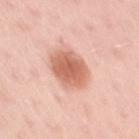Captured during whole-body skin photography for melanoma surveillance; the lesion was not biopsied.
Cropped from a whole-body photographic skin survey; the tile spans about 15 mm.
On the right upper arm.
The patient is a female aged around 50.